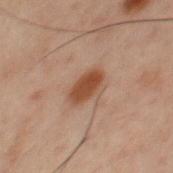Automated image analysis of the tile measured a lesion color around L≈35 a*≈18 b*≈25 in CIELAB, roughly 10 lightness units darker than nearby skin, and a normalized border contrast of about 9.5. It also reported a border-irregularity rating of about 2/10 and a within-lesion color-variation index near 1.5/10. The software also gave a nevus-likeness score of about 95/100 and a detector confidence of about 100 out of 100 that the crop contains a lesion.
Located on the chest.
This is a cross-polarized tile.
A male patient about 60 years old.
A roughly 15 mm field-of-view crop from a total-body skin photograph.